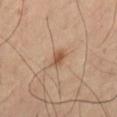biopsy status: imaged on a skin check; not biopsied | TBP lesion metrics: an outline eccentricity of about 0.6 (0 = round, 1 = elongated) and two-axis asymmetry of about 0.3; a lesion–skin lightness drop of about 10 and a lesion-to-skin contrast of about 8 (normalized; higher = more distinct); a border-irregularity rating of about 2.5/10 and internal color variation of about 3.5 on a 0–10 scale | size: ≈2 mm | subject: male, about 60 years old | anatomic site: the abdomen | acquisition: 15 mm crop, total-body photography.